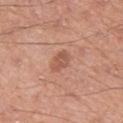Impression: This lesion was catalogued during total-body skin photography and was not selected for biopsy.